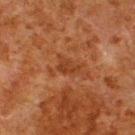The lesion was photographed on a routine skin check and not biopsied; there is no pathology result. A male patient aged around 80. This image is a 15 mm lesion crop taken from a total-body photograph. Imaged with cross-polarized lighting. On the upper back.